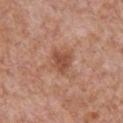* biopsy status — no biopsy performed (imaged during a skin exam)
* anatomic site — the chest
* subject — male, about 65 years old
* image — ~15 mm tile from a whole-body skin photo